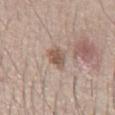The subject is a male aged 38 to 42. This is a white-light tile. A 15 mm close-up extracted from a 3D total-body photography capture. Longest diameter approximately 3.5 mm. Located on the abdomen.